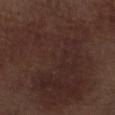This lesion was catalogued during total-body skin photography and was not selected for biopsy. A male patient aged around 70. The lesion is located on the right thigh. Cropped from a total-body skin-imaging series; the visible field is about 15 mm. The lesion's longest dimension is about 20 mm. Automated tile analysis of the lesion measured two-axis asymmetry of about 0.55.